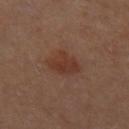biopsy status: total-body-photography surveillance lesion; no biopsy
imaging modality: 15 mm crop, total-body photography
body site: the right thigh
subject: female, aged 48–52